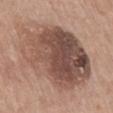Part of a total-body skin-imaging series; this lesion was reviewed on a skin check and was not flagged for biopsy.
Located on the mid back.
The subject is a female in their 60s.
Cropped from a total-body skin-imaging series; the visible field is about 15 mm.